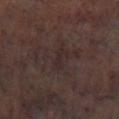Recorded during total-body skin imaging; not selected for excision or biopsy.
Imaged with cross-polarized lighting.
The lesion is on the left lower leg.
The subject is a male in their mid- to late 60s.
A 15 mm close-up extracted from a 3D total-body photography capture.
Longest diameter approximately 3 mm.
An algorithmic analysis of the crop reported lesion-presence confidence of about 65/100.